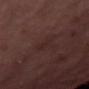Notes:
– biopsy status: imaged on a skin check; not biopsied
– illumination: cross-polarized
– subject: female, about 55 years old
– image: total-body-photography crop, ~15 mm field of view
– location: the right thigh
– image-analysis metrics: an area of roughly 2.5 mm², a shape eccentricity near 0.95, and two-axis asymmetry of about 0.3
– diameter: ~3 mm (longest diameter)